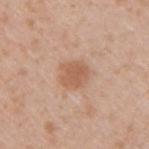Q: Who is the patient?
A: male, roughly 35 years of age
Q: Lesion location?
A: the right upper arm
Q: What kind of image is this?
A: ~15 mm tile from a whole-body skin photo